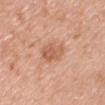- notes: total-body-photography surveillance lesion; no biopsy
- automated metrics: a lesion–skin lightness drop of about 10 and a lesion-to-skin contrast of about 6.5 (normalized; higher = more distinct); a border-irregularity rating of about 2.5/10 and internal color variation of about 3 on a 0–10 scale
- image: total-body-photography crop, ~15 mm field of view
- illumination: white-light
- diameter: ~3.5 mm (longest diameter)
- patient: male, in their 40s
- location: the front of the torso